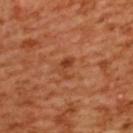biopsy_status: not biopsied; imaged during a skin examination
site: upper back
automated_metrics:
  area_mm2_approx: 2.5
  eccentricity: 0.85
  shape_asymmetry: 0.55
  cielab_L: 43
  cielab_a: 30
  cielab_b: 38
  vs_skin_darker_L: 9.0
  vs_skin_contrast_norm: 7.0
image:
  source: total-body photography crop
  field_of_view_mm: 15
lighting: cross-polarized
lesion_size:
  long_diameter_mm_approx: 2.5
patient:
  sex: female
  age_approx: 55